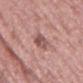The lesion was photographed on a routine skin check and not biopsied; there is no pathology result. The subject is a female approximately 40 years of age. Cropped from a total-body skin-imaging series; the visible field is about 15 mm. Automated image analysis of the tile measured a footprint of about 5.5 mm², an outline eccentricity of about 0.8 (0 = round, 1 = elongated), and two-axis asymmetry of about 0.25. The software also gave a lesion color around L≈52 a*≈23 b*≈22 in CIELAB and a normalized lesion–skin contrast near 8. The software also gave border irregularity of about 2.5 on a 0–10 scale and internal color variation of about 4.5 on a 0–10 scale. Located on the left thigh.